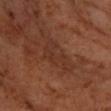biopsy_status: not biopsied; imaged during a skin examination
image:
  source: total-body photography crop
  field_of_view_mm: 15
lesion_size:
  long_diameter_mm_approx: 5.0
lighting: cross-polarized
site: right forearm
patient:
  sex: male
  age_approx: 60